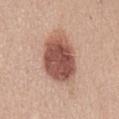Findings:
• biopsy status · catalogued during a skin exam; not biopsied
• subject · female, roughly 55 years of age
• site · the front of the torso
• acquisition · ~15 mm crop, total-body skin-cancer survey